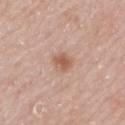Impression: No biopsy was performed on this lesion — it was imaged during a full skin examination and was not determined to be concerning. Background: The lesion-visualizer software estimated a footprint of about 5 mm², an eccentricity of roughly 0.75, and a shape-asymmetry score of about 0.25 (0 = symmetric). And it measured a lesion color around L≈58 a*≈21 b*≈29 in CIELAB, about 10 CIELAB-L* units darker than the surrounding skin, and a normalized lesion–skin contrast near 7. The analysis additionally found an automated nevus-likeness rating near 75 out of 100 and lesion-presence confidence of about 100/100. From the mid back. A male patient aged 63–67. A close-up tile cropped from a whole-body skin photograph, about 15 mm across. Longest diameter approximately 3 mm. The tile uses white-light illumination.